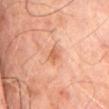Q: Is there a histopathology result?
A: catalogued during a skin exam; not biopsied
Q: Lesion location?
A: the back
Q: What lighting was used for the tile?
A: cross-polarized
Q: Who is the patient?
A: male, aged 63 to 67
Q: What kind of image is this?
A: total-body-photography crop, ~15 mm field of view